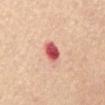Recorded during total-body skin imaging; not selected for excision or biopsy.